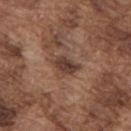<lesion>
<biopsy_status>not biopsied; imaged during a skin examination</biopsy_status>
<image>
  <source>total-body photography crop</source>
  <field_of_view_mm>15</field_of_view_mm>
</image>
<site>upper back</site>
<patient>
  <sex>male</sex>
  <age_approx>75</age_approx>
</patient>
<lighting>white-light</lighting>
<lesion_size>
  <long_diameter_mm_approx>3.5</long_diameter_mm_approx>
</lesion_size>
<automated_metrics>
  <area_mm2_approx>5.0</area_mm2_approx>
  <shape_asymmetry>0.3</shape_asymmetry>
  <border_irregularity_0_10>3.0</border_irregularity_0_10>
  <peripheral_color_asymmetry>1.0</peripheral_color_asymmetry>
</automated_metrics>
</lesion>